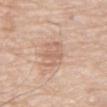Impression:
Imaged during a routine full-body skin examination; the lesion was not biopsied and no histopathology is available.
Background:
An algorithmic analysis of the crop reported a footprint of about 9 mm², an eccentricity of roughly 0.65, and a symmetry-axis asymmetry near 0.25. The software also gave an average lesion color of about L≈64 a*≈19 b*≈29 (CIELAB), a lesion–skin lightness drop of about 7, and a normalized border contrast of about 5. The analysis additionally found a within-lesion color-variation index near 3/10 and peripheral color asymmetry of about 1. The analysis additionally found an automated nevus-likeness rating near 0 out of 100 and lesion-presence confidence of about 100/100. The lesion is on the mid back. The subject is a male aged approximately 80. A 15 mm close-up tile from a total-body photography series done for melanoma screening. Approximately 4 mm at its widest.